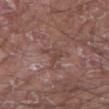Clinical impression: The lesion was photographed on a routine skin check and not biopsied; there is no pathology result. Image and clinical context: Captured under white-light illumination. Cropped from a total-body skin-imaging series; the visible field is about 15 mm. The recorded lesion diameter is about 2.5 mm. The patient is a male aged 63–67. From the right upper arm.